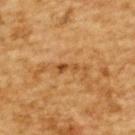Q: Was this lesion biopsied?
A: catalogued during a skin exam; not biopsied
Q: Automated lesion metrics?
A: an area of roughly 2.5 mm², an outline eccentricity of about 0.95 (0 = round, 1 = elongated), and a symmetry-axis asymmetry near 0.4; border irregularity of about 5 on a 0–10 scale, internal color variation of about 0 on a 0–10 scale, and a peripheral color-asymmetry measure near 0
Q: What lighting was used for the tile?
A: cross-polarized illumination
Q: What are the patient's age and sex?
A: male, aged approximately 85 or older
Q: What is the imaging modality?
A: ~15 mm crop, total-body skin-cancer survey
Q: Lesion size?
A: ≈2.5 mm
Q: What is the anatomic site?
A: the upper back